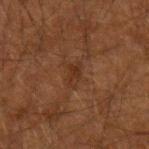Impression:
No biopsy was performed on this lesion — it was imaged during a full skin examination and was not determined to be concerning.
Image and clinical context:
Automated image analysis of the tile measured a footprint of about 3.5 mm², an outline eccentricity of about 0.8 (0 = round, 1 = elongated), and a symmetry-axis asymmetry near 0.35. The software also gave a lesion color around L≈24 a*≈16 b*≈24 in CIELAB, roughly 5 lightness units darker than nearby skin, and a normalized lesion–skin contrast near 6. Located on the left forearm. Cropped from a whole-body photographic skin survey; the tile spans about 15 mm. Imaged with cross-polarized lighting. A male patient, aged 48–52.